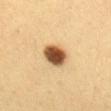biopsy status = catalogued during a skin exam; not biopsied | acquisition = total-body-photography crop, ~15 mm field of view | anatomic site = the mid back | subject = female, aged 28–32.